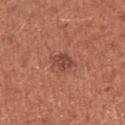notes = catalogued during a skin exam; not biopsied
patient = female, aged approximately 35
size = ≈2.5 mm
anatomic site = the right upper arm
TBP lesion metrics = a footprint of about 4.5 mm², a shape eccentricity near 0.65, and two-axis asymmetry of about 0.25
acquisition = 15 mm crop, total-body photography